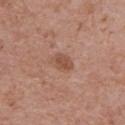follow-up=no biopsy performed (imaged during a skin exam) | imaging modality=total-body-photography crop, ~15 mm field of view | site=the chest | size=≈3 mm | automated metrics=a footprint of about 4.5 mm², an eccentricity of roughly 0.75, and a symmetry-axis asymmetry near 0.25; an average lesion color of about L≈51 a*≈22 b*≈29 (CIELAB), roughly 9 lightness units darker than nearby skin, and a normalized border contrast of about 6.5; a classifier nevus-likeness of about 50/100 and a detector confidence of about 100 out of 100 that the crop contains a lesion | tile lighting=white-light | subject=male, about 70 years old.